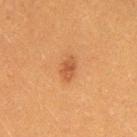Clinical impression:
Recorded during total-body skin imaging; not selected for excision or biopsy.
Acquisition and patient details:
Longest diameter approximately 3 mm. A female subject aged 38–42. An algorithmic analysis of the crop reported an area of roughly 4 mm², an outline eccentricity of about 0.75 (0 = round, 1 = elongated), and a shape-asymmetry score of about 0.15 (0 = symmetric). The analysis additionally found an average lesion color of about L≈45 a*≈23 b*≈34 (CIELAB), roughly 8 lightness units darker than nearby skin, and a lesion-to-skin contrast of about 6.5 (normalized; higher = more distinct). Located on the left thigh. A 15 mm crop from a total-body photograph taken for skin-cancer surveillance.